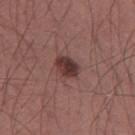Acquisition and patient details: The lesion-visualizer software estimated an average lesion color of about L≈35 a*≈21 b*≈19 (CIELAB), about 13 CIELAB-L* units darker than the surrounding skin, and a lesion-to-skin contrast of about 11 (normalized; higher = more distinct). Longest diameter approximately 3 mm. The lesion is on the right thigh. A region of skin cropped from a whole-body photographic capture, roughly 15 mm wide. A male subject, in their mid- to late 40s.